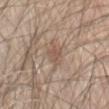A 15 mm close-up tile from a total-body photography series done for melanoma screening. From the abdomen. The tile uses white-light illumination. A male patient approximately 80 years of age.